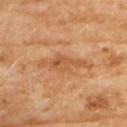No biopsy was performed on this lesion — it was imaged during a full skin examination and was not determined to be concerning. The tile uses cross-polarized illumination. A female subject, approximately 60 years of age. A 15 mm close-up tile from a total-body photography series done for melanoma screening. The lesion is located on the upper back. Measured at roughly 6 mm in maximum diameter.